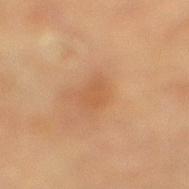Context:
The lesion's longest dimension is about 3 mm. A region of skin cropped from a whole-body photographic capture, roughly 15 mm wide. This is a cross-polarized tile. Located on the right lower leg. The subject is a male aged around 85 or older.